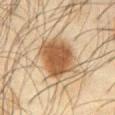Clinical impression: This lesion was catalogued during total-body skin photography and was not selected for biopsy. Clinical summary: The total-body-photography lesion software estimated a footprint of about 18 mm², a shape eccentricity near 0.4, and two-axis asymmetry of about 0.15. The analysis additionally found a mean CIELAB color near L≈45 a*≈16 b*≈31 and a lesion–skin lightness drop of about 13. The analysis additionally found a within-lesion color-variation index near 3.5/10. It also reported a nevus-likeness score of about 100/100. The lesion is located on the abdomen. A male patient approximately 65 years of age. A roughly 15 mm field-of-view crop from a total-body skin photograph. Imaged with cross-polarized lighting. About 5 mm across.